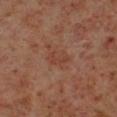Impression:
This lesion was catalogued during total-body skin photography and was not selected for biopsy.
Image and clinical context:
An algorithmic analysis of the crop reported an average lesion color of about L≈38 a*≈22 b*≈27 (CIELAB) and about 5 CIELAB-L* units darker than the surrounding skin. The software also gave a border-irregularity rating of about 3.5/10, a color-variation rating of about 1/10, and radial color variation of about 0.5. The software also gave an automated nevus-likeness rating near 0 out of 100. From the left lower leg. A male subject, about 60 years old. The lesion's longest dimension is about 3 mm. A lesion tile, about 15 mm wide, cut from a 3D total-body photograph.